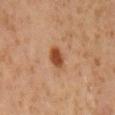| field | value |
|---|---|
| follow-up | no biopsy performed (imaged during a skin exam) |
| tile lighting | cross-polarized |
| patient | male, in their 60s |
| size | ≈3 mm |
| body site | the mid back |
| acquisition | 15 mm crop, total-body photography |